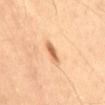Part of a total-body skin-imaging series; this lesion was reviewed on a skin check and was not flagged for biopsy.
The total-body-photography lesion software estimated an area of roughly 3.5 mm², an outline eccentricity of about 0.9 (0 = round, 1 = elongated), and two-axis asymmetry of about 0.2. And it measured lesion-presence confidence of about 100/100.
The tile uses cross-polarized illumination.
The recorded lesion diameter is about 3 mm.
A male patient, roughly 40 years of age.
The lesion is on the abdomen.
This image is a 15 mm lesion crop taken from a total-body photograph.